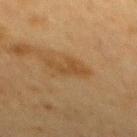Notes:
• notes: catalogued during a skin exam; not biopsied
• lighting: cross-polarized illumination
• body site: the mid back
• image-analysis metrics: border irregularity of about 5 on a 0–10 scale, a within-lesion color-variation index near 1/10, and a peripheral color-asymmetry measure near 0.5
• acquisition: ~15 mm tile from a whole-body skin photo
• subject: male, aged 58 to 62